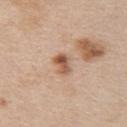This lesion was catalogued during total-body skin photography and was not selected for biopsy.
A female subject, aged approximately 45.
From the chest.
About 3 mm across.
A 15 mm crop from a total-body photograph taken for skin-cancer surveillance.